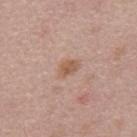biopsy_status: not biopsied; imaged during a skin examination
image:
  source: total-body photography crop
  field_of_view_mm: 15
patient:
  sex: male
  age_approx: 45
lighting: white-light
site: upper back
lesion_size:
  long_diameter_mm_approx: 3.0